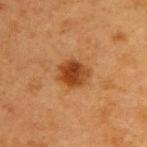  biopsy_status: not biopsied; imaged during a skin examination
  image:
    source: total-body photography crop
    field_of_view_mm: 15
  site: right upper arm
  patient:
    sex: female
    age_approx: 40
  automated_metrics:
    eccentricity: 0.45
    shape_asymmetry: 0.15
    nevus_likeness_0_100: 100
    lesion_detection_confidence_0_100: 100
  lighting: cross-polarized